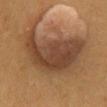On the mid back.
The lesion's longest dimension is about 8.5 mm.
The lesion-visualizer software estimated an area of roughly 38 mm² and two-axis asymmetry of about 0.45. It also reported border irregularity of about 5.5 on a 0–10 scale.
This is a cross-polarized tile.
The patient is a male in their mid- to late 50s.
A roughly 15 mm field-of-view crop from a total-body skin photograph.